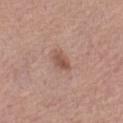Findings:
- biopsy status: no biopsy performed (imaged during a skin exam)
- body site: the left thigh
- size: ≈2.5 mm
- patient: female, aged 58 to 62
- tile lighting: white-light
- image source: ~15 mm tile from a whole-body skin photo